From the upper back · a male patient aged around 60 · a region of skin cropped from a whole-body photographic capture, roughly 15 mm wide — 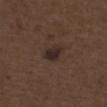Findings:
* automated metrics — a footprint of about 4.5 mm², an eccentricity of roughly 0.7, and two-axis asymmetry of about 0.3; a mean CIELAB color near L≈25 a*≈14 b*≈18, about 8 CIELAB-L* units darker than the surrounding skin, and a normalized border contrast of about 10; border irregularity of about 2.5 on a 0–10 scale, a within-lesion color-variation index near 2.5/10, and peripheral color asymmetry of about 1
* tile lighting — white-light
* diagnosis — an atypical melanocytic neoplasm (borderline)